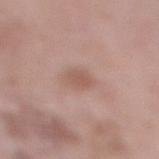Impression:
Imaged during a routine full-body skin examination; the lesion was not biopsied and no histopathology is available.
Background:
From the right lower leg. Automated tile analysis of the lesion measured an eccentricity of roughly 0.85 and a shape-asymmetry score of about 0.2 (0 = symmetric). The analysis additionally found a mean CIELAB color near L≈55 a*≈20 b*≈26 and a normalized border contrast of about 6. And it measured a border-irregularity rating of about 2/10, a within-lesion color-variation index near 1/10, and peripheral color asymmetry of about 0.5. Measured at roughly 3 mm in maximum diameter. A lesion tile, about 15 mm wide, cut from a 3D total-body photograph. Imaged with white-light lighting. A female subject about 70 years old.